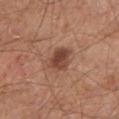Part of a total-body skin-imaging series; this lesion was reviewed on a skin check and was not flagged for biopsy.
Cropped from a total-body skin-imaging series; the visible field is about 15 mm.
The tile uses white-light illumination.
A male patient aged 63 to 67.
The lesion-visualizer software estimated a footprint of about 7 mm² and an eccentricity of roughly 0.65. It also reported border irregularity of about 2.5 on a 0–10 scale, a within-lesion color-variation index near 4.5/10, and radial color variation of about 1.5.
From the chest.
About 3.5 mm across.